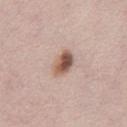Case summary:
* workup — total-body-photography surveillance lesion; no biopsy
* lesion diameter — ~3.5 mm (longest diameter)
* patient — male, approximately 30 years of age
* image-analysis metrics — an eccentricity of roughly 0.75 and a shape-asymmetry score of about 0.15 (0 = symmetric)
* acquisition — ~15 mm crop, total-body skin-cancer survey
* illumination — white-light